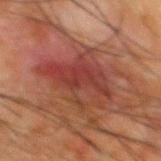{"biopsy_status": "not biopsied; imaged during a skin examination", "image": {"source": "total-body photography crop", "field_of_view_mm": 15}, "site": "back", "patient": {"sex": "male", "age_approx": 65}}